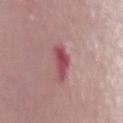Findings:
- subject — female, in their 50s
- location — the chest
- image source — ~15 mm tile from a whole-body skin photo